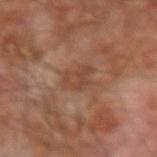This lesion was catalogued during total-body skin photography and was not selected for biopsy.
This is a cross-polarized tile.
Located on the right forearm.
About 3.5 mm across.
Automated tile analysis of the lesion measured a border-irregularity index near 3.5/10, internal color variation of about 2.5 on a 0–10 scale, and radial color variation of about 1. The software also gave a classifier nevus-likeness of about 0/100.
A roughly 15 mm field-of-view crop from a total-body skin photograph.
A male subject aged approximately 65.